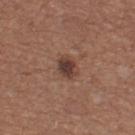{"site": "left thigh", "patient": {"sex": "female", "age_approx": 35}, "image": {"source": "total-body photography crop", "field_of_view_mm": 15}}